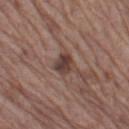Captured during whole-body skin photography for melanoma surveillance; the lesion was not biopsied. The total-body-photography lesion software estimated an average lesion color of about L≈39 a*≈18 b*≈21 (CIELAB), a lesion–skin lightness drop of about 11, and a normalized border contrast of about 9.5. It also reported a nevus-likeness score of about 5/100 and a lesion-detection confidence of about 100/100. Located on the leg. This image is a 15 mm lesion crop taken from a total-body photograph. Approximately 2.5 mm at its widest. The subject is a male about 65 years old.